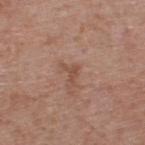| feature | finding |
|---|---|
| notes | total-body-photography surveillance lesion; no biopsy |
| subject | male, approximately 55 years of age |
| tile lighting | white-light |
| lesion size | ~2.5 mm (longest diameter) |
| anatomic site | the upper back |
| acquisition | ~15 mm crop, total-body skin-cancer survey |